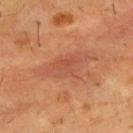follow-up — total-body-photography surveillance lesion; no biopsy
automated metrics — a lesion area of about 12 mm², an outline eccentricity of about 0.85 (0 = round, 1 = elongated), and a symmetry-axis asymmetry near 0.3; a mean CIELAB color near L≈44 a*≈24 b*≈30 and about 6 CIELAB-L* units darker than the surrounding skin; border irregularity of about 4 on a 0–10 scale, a within-lesion color-variation index near 3/10, and peripheral color asymmetry of about 1
acquisition — total-body-photography crop, ~15 mm field of view
lighting — cross-polarized illumination
site — the chest
size — ~5.5 mm (longest diameter)
subject — male, aged around 55